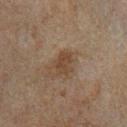notes = no biopsy performed (imaged during a skin exam) | tile lighting = cross-polarized illumination | automated lesion analysis = an average lesion color of about L≈31 a*≈12 b*≈23 (CIELAB), about 6 CIELAB-L* units darker than the surrounding skin, and a normalized lesion–skin contrast near 7; border irregularity of about 2.5 on a 0–10 scale, a color-variation rating of about 1.5/10, and peripheral color asymmetry of about 0.5 | acquisition = 15 mm crop, total-body photography | diameter = ~2.5 mm (longest diameter) | body site = the left lower leg | patient = male, aged around 60.